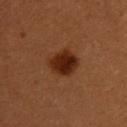A female subject approximately 40 years of age. Imaged with cross-polarized lighting. Cropped from a total-body skin-imaging series; the visible field is about 15 mm. The lesion's longest dimension is about 3.5 mm. The lesion is on the upper back.